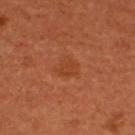Q: How was the tile lit?
A: cross-polarized illumination
Q: What is the imaging modality?
A: 15 mm crop, total-body photography
Q: Lesion size?
A: ~3 mm (longest diameter)
Q: Patient demographics?
A: female, aged around 50